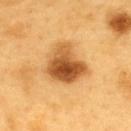workup: no biopsy performed (imaged during a skin exam)
subject: male, in their 60s
image: 15 mm crop, total-body photography
location: the upper back
lighting: cross-polarized
lesion size: ~5.5 mm (longest diameter)